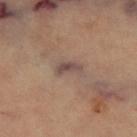| key | value |
|---|---|
| workup | imaged on a skin check; not biopsied |
| image | 15 mm crop, total-body photography |
| patient | female, about 70 years old |
| location | the left thigh |